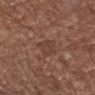• workup · catalogued during a skin exam; not biopsied
• site · the right forearm
• TBP lesion metrics · an eccentricity of roughly 0.7; a border-irregularity index near 2/10, a color-variation rating of about 2.5/10, and a peripheral color-asymmetry measure near 1; an automated nevus-likeness rating near 0 out of 100 and a lesion-detection confidence of about 75/100
• imaging modality · ~15 mm crop, total-body skin-cancer survey
• size · about 3 mm
• lighting · white-light
• subject · male, about 75 years old